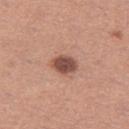Clinical impression: Captured during whole-body skin photography for melanoma surveillance; the lesion was not biopsied. Acquisition and patient details: The lesion is on the left thigh. The tile uses white-light illumination. Longest diameter approximately 3 mm. A lesion tile, about 15 mm wide, cut from a 3D total-body photograph. A female subject aged around 30.